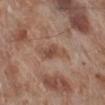The lesion was photographed on a routine skin check and not biopsied; there is no pathology result. The lesion's longest dimension is about 4.5 mm. The subject is a male in their 70s. A roughly 15 mm field-of-view crop from a total-body skin photograph. Located on the right lower leg. Imaged with white-light lighting.